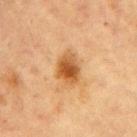Clinical impression: No biopsy was performed on this lesion — it was imaged during a full skin examination and was not determined to be concerning. Clinical summary: The total-body-photography lesion software estimated a border-irregularity index near 1.5/10 and a within-lesion color-variation index near 5.5/10. It also reported a nevus-likeness score of about 90/100. The recorded lesion diameter is about 3.5 mm. Cropped from a total-body skin-imaging series; the visible field is about 15 mm. A female patient, aged around 65. The tile uses cross-polarized illumination. The lesion is on the right upper arm.